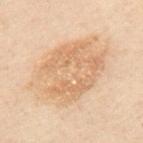Captured during whole-body skin photography for melanoma surveillance; the lesion was not biopsied.
The lesion is located on the mid back.
Imaged with cross-polarized lighting.
The lesion's longest dimension is about 10.5 mm.
An algorithmic analysis of the crop reported a footprint of about 48 mm², an eccentricity of roughly 0.85, and a symmetry-axis asymmetry near 0.15. The analysis additionally found a mean CIELAB color near L≈57 a*≈14 b*≈30 and roughly 7 lightness units darker than nearby skin. It also reported a nevus-likeness score of about 5/100 and lesion-presence confidence of about 100/100.
A male patient aged 48–52.
This image is a 15 mm lesion crop taken from a total-body photograph.